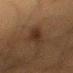Imaged during a routine full-body skin examination; the lesion was not biopsied and no histopathology is available.
Captured under cross-polarized illumination.
A roughly 15 mm field-of-view crop from a total-body skin photograph.
A male subject, aged 48–52.
The lesion's longest dimension is about 4.5 mm.
Located on the left upper arm.